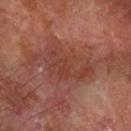{"biopsy_status": "not biopsied; imaged during a skin examination", "lesion_size": {"long_diameter_mm_approx": 6.5}, "site": "left forearm", "image": {"source": "total-body photography crop", "field_of_view_mm": 15}, "patient": {"sex": "male", "age_approx": 70}}